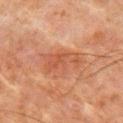{"image": {"source": "total-body photography crop", "field_of_view_mm": 15}, "patient": {"sex": "male", "age_approx": 65}, "site": "right upper arm", "lighting": "cross-polarized", "lesion_size": {"long_diameter_mm_approx": 5.0}}